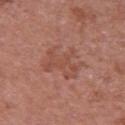<lesion>
<biopsy_status>not biopsied; imaged during a skin examination</biopsy_status>
<site>right upper arm</site>
<lighting>white-light</lighting>
<patient>
  <sex>male</sex>
  <age_approx>65</age_approx>
</patient>
<image>
  <source>total-body photography crop</source>
  <field_of_view_mm>15</field_of_view_mm>
</image>
</lesion>